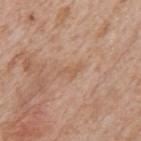acquisition — total-body-photography crop, ~15 mm field of view
tile lighting — white-light
subject — male, about 65 years old
lesion diameter — ≈3 mm
site — the mid back